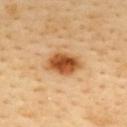follow-up — catalogued during a skin exam; not biopsied | illumination — cross-polarized | location — the upper back | size — ~4.5 mm (longest diameter) | acquisition — total-body-photography crop, ~15 mm field of view | subject — female, aged 48 to 52.